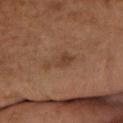Recorded during total-body skin imaging; not selected for excision or biopsy. The lesion is on the arm. A female patient, aged 68–72. A 15 mm close-up tile from a total-body photography series done for melanoma screening.